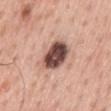Captured during whole-body skin photography for melanoma surveillance; the lesion was not biopsied. A region of skin cropped from a whole-body photographic capture, roughly 15 mm wide. A male subject, aged 58–62. The lesion is on the mid back. Measured at roughly 4 mm in maximum diameter.